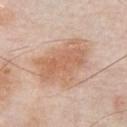  biopsy_status: not biopsied; imaged during a skin examination
  patient:
    sex: male
    age_approx: 55
  image:
    source: total-body photography crop
    field_of_view_mm: 15
  lighting: white-light
  site: chest